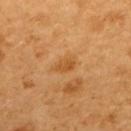The lesion was photographed on a routine skin check and not biopsied; there is no pathology result.
A female subject approximately 55 years of age.
A lesion tile, about 15 mm wide, cut from a 3D total-body photograph.
The lesion is on the upper back.